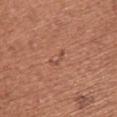Recorded during total-body skin imaging; not selected for excision or biopsy.